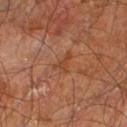Assessment: Captured during whole-body skin photography for melanoma surveillance; the lesion was not biopsied. Context: Cropped from a whole-body photographic skin survey; the tile spans about 15 mm. A male patient, approximately 60 years of age. The recorded lesion diameter is about 3 mm. From the leg.